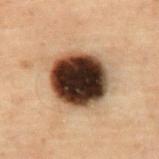Q: Lesion location?
A: the abdomen
Q: Automated lesion metrics?
A: a footprint of about 28 mm², an eccentricity of roughly 0.4, and two-axis asymmetry of about 0.1; a mean CIELAB color near L≈26 a*≈13 b*≈20 and about 26 CIELAB-L* units darker than the surrounding skin
Q: Who is the patient?
A: male, about 70 years old
Q: Illumination type?
A: cross-polarized illumination
Q: How was this image acquired?
A: total-body-photography crop, ~15 mm field of view
Q: What is the lesion's diameter?
A: ≈6.5 mm